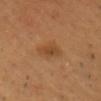site = the head or neck
subject = male, about 60 years old
imaging modality = ~15 mm crop, total-body skin-cancer survey